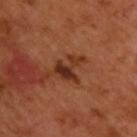| feature | finding |
|---|---|
| follow-up | total-body-photography surveillance lesion; no biopsy |
| anatomic site | the upper back |
| imaging modality | total-body-photography crop, ~15 mm field of view |
| lighting | cross-polarized illumination |
| patient | male, aged 48 to 52 |